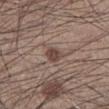{
  "biopsy_status": "not biopsied; imaged during a skin examination",
  "image": {
    "source": "total-body photography crop",
    "field_of_view_mm": 15
  },
  "lighting": "white-light",
  "patient": {
    "sex": "male",
    "age_approx": 35
  },
  "lesion_size": {
    "long_diameter_mm_approx": 2.5
  },
  "site": "left lower leg"
}